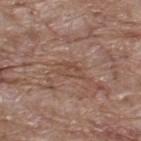Q: Was a biopsy performed?
A: no biopsy performed (imaged during a skin exam)
Q: How large is the lesion?
A: about 3.5 mm
Q: Where on the body is the lesion?
A: the upper back
Q: Automated lesion metrics?
A: a lesion area of about 4.5 mm², an outline eccentricity of about 0.8 (0 = round, 1 = elongated), and a shape-asymmetry score of about 0.35 (0 = symmetric); border irregularity of about 3.5 on a 0–10 scale, internal color variation of about 2.5 on a 0–10 scale, and a peripheral color-asymmetry measure near 1; a classifier nevus-likeness of about 0/100 and lesion-presence confidence of about 55/100
Q: Patient demographics?
A: male, about 70 years old
Q: What lighting was used for the tile?
A: white-light illumination
Q: What is the imaging modality?
A: ~15 mm crop, total-body skin-cancer survey